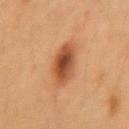The lesion was photographed on a routine skin check and not biopsied; there is no pathology result. The lesion is on the arm. A close-up tile cropped from a whole-body skin photograph, about 15 mm across. Captured under cross-polarized illumination. An algorithmic analysis of the crop reported a border-irregularity rating of about 1.5/10 and a within-lesion color-variation index near 5.5/10. The software also gave an automated nevus-likeness rating near 100 out of 100 and a lesion-detection confidence of about 100/100. The recorded lesion diameter is about 4.5 mm. A female patient, aged 38 to 42.